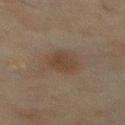Context: The total-body-photography lesion software estimated a lesion area of about 8.5 mm², a shape eccentricity near 0.55, and a symmetry-axis asymmetry near 0.15. It also reported border irregularity of about 1.5 on a 0–10 scale, internal color variation of about 1.5 on a 0–10 scale, and radial color variation of about 0.5. The analysis additionally found a classifier nevus-likeness of about 90/100. From the chest. Longest diameter approximately 3.5 mm. Imaged with cross-polarized lighting. A male patient, aged around 70. A 15 mm close-up extracted from a 3D total-body photography capture.